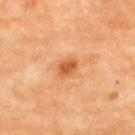  biopsy_status: not biopsied; imaged during a skin examination
  site: upper back
  patient:
    age_approx: 65
  image:
    source: total-body photography crop
    field_of_view_mm: 15